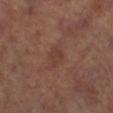notes: catalogued during a skin exam; not biopsied | patient: male, aged around 65 | acquisition: ~15 mm crop, total-body skin-cancer survey | body site: the right lower leg.